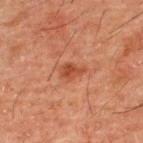| field | value |
|---|---|
| workup | no biopsy performed (imaged during a skin exam) |
| subject | male, aged 48 to 52 |
| imaging modality | 15 mm crop, total-body photography |
| site | the upper back |
| illumination | cross-polarized illumination |
| size | ~3 mm (longest diameter) |
| automated lesion analysis | a footprint of about 4 mm², an eccentricity of roughly 0.8, and a shape-asymmetry score of about 0.3 (0 = symmetric); a lesion–skin lightness drop of about 8 and a normalized border contrast of about 7 |